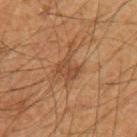Assessment: The lesion was tiled from a total-body skin photograph and was not biopsied. Image and clinical context: A male patient aged approximately 65. The lesion is located on the arm. Cropped from a total-body skin-imaging series; the visible field is about 15 mm.